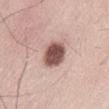Q: Lesion location?
A: the front of the torso
Q: How was the tile lit?
A: white-light illumination
Q: What is the imaging modality?
A: ~15 mm crop, total-body skin-cancer survey
Q: Lesion size?
A: ≈3.5 mm
Q: What are the patient's age and sex?
A: male, aged approximately 35